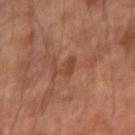| key | value |
|---|---|
| subject | male, aged around 60 |
| lesion size | about 2.5 mm |
| location | the right forearm |
| automated lesion analysis | a classifier nevus-likeness of about 0/100 |
| acquisition | ~15 mm crop, total-body skin-cancer survey |
| illumination | cross-polarized |